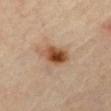Case summary:
- image · 15 mm crop, total-body photography
- site · the abdomen
- subject · male, aged 63–67